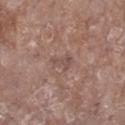biopsy_status: not biopsied; imaged during a skin examination
image:
  source: total-body photography crop
  field_of_view_mm: 15
patient:
  sex: female
  age_approx: 75
site: leg